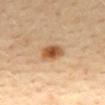This lesion was catalogued during total-body skin photography and was not selected for biopsy. Located on the mid back. The total-body-photography lesion software estimated a nevus-likeness score of about 100/100. This image is a 15 mm lesion crop taken from a total-body photograph. Longest diameter approximately 3.5 mm. Captured under cross-polarized illumination. A female patient, about 45 years old.